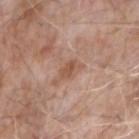An algorithmic analysis of the crop reported a mean CIELAB color near L≈53 a*≈21 b*≈29. It also reported a border-irregularity rating of about 3/10, internal color variation of about 2 on a 0–10 scale, and radial color variation of about 0.5. It also reported a classifier nevus-likeness of about 0/100 and a detector confidence of about 100 out of 100 that the crop contains a lesion. The recorded lesion diameter is about 2.5 mm. A male patient, in their mid-70s. The tile uses white-light illumination. The lesion is on the right forearm. A 15 mm crop from a total-body photograph taken for skin-cancer surveillance.